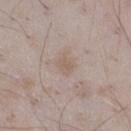Impression:
This lesion was catalogued during total-body skin photography and was not selected for biopsy.
Background:
The tile uses white-light illumination. About 2.5 mm across. The lesion is on the right thigh. A 15 mm crop from a total-body photograph taken for skin-cancer surveillance. The lesion-visualizer software estimated a lesion area of about 3.5 mm² and a shape eccentricity near 0.75. And it measured a border-irregularity index near 2.5/10 and a peripheral color-asymmetry measure near 0.5. A male patient, aged 43–47.